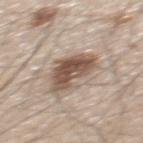Part of a total-body skin-imaging series; this lesion was reviewed on a skin check and was not flagged for biopsy.
A lesion tile, about 15 mm wide, cut from a 3D total-body photograph.
A male patient aged around 60.
Located on the back.